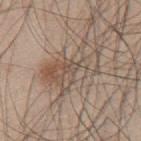workup: total-body-photography surveillance lesion; no biopsy | body site: the upper back | patient: male, in their mid- to late 40s | illumination: white-light illumination | imaging modality: total-body-photography crop, ~15 mm field of view | diameter: ≈7.5 mm.